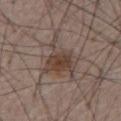Q: Was a biopsy performed?
A: imaged on a skin check; not biopsied
Q: Lesion size?
A: about 5.5 mm
Q: What are the patient's age and sex?
A: male, roughly 80 years of age
Q: What is the imaging modality?
A: total-body-photography crop, ~15 mm field of view
Q: What did automated image analysis measure?
A: an average lesion color of about L≈42 a*≈14 b*≈22 (CIELAB); a color-variation rating of about 4/10; a nevus-likeness score of about 100/100
Q: How was the tile lit?
A: white-light
Q: What is the anatomic site?
A: the front of the torso